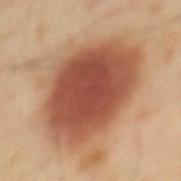A 15 mm close-up extracted from a 3D total-body photography capture. The lesion is located on the mid back. A male patient, aged approximately 40. This is a cross-polarized tile.